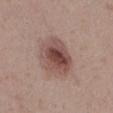image-analysis metrics = a mean CIELAB color near L≈48 a*≈20 b*≈22 and a lesion–skin lightness drop of about 12; an automated nevus-likeness rating near 95 out of 100 and lesion-presence confidence of about 100/100
acquisition = 15 mm crop, total-body photography
anatomic site = the left thigh
patient = female, aged approximately 30
size = ≈4.5 mm
tile lighting = white-light illumination